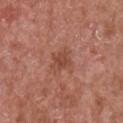Clinical impression: Recorded during total-body skin imaging; not selected for excision or biopsy. Image and clinical context: This image is a 15 mm lesion crop taken from a total-body photograph. Captured under white-light illumination. Located on the right upper arm. A male patient in their mid- to late 60s. An algorithmic analysis of the crop reported an average lesion color of about L≈47 a*≈25 b*≈29 (CIELAB), about 8 CIELAB-L* units darker than the surrounding skin, and a lesion-to-skin contrast of about 6 (normalized; higher = more distinct). The analysis additionally found a classifier nevus-likeness of about 10/100 and a detector confidence of about 100 out of 100 that the crop contains a lesion.